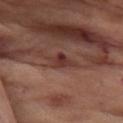Q: Was this lesion biopsied?
A: total-body-photography surveillance lesion; no biopsy
Q: What kind of image is this?
A: 15 mm crop, total-body photography
Q: What is the anatomic site?
A: the front of the torso
Q: What is the lesion's diameter?
A: ~4 mm (longest diameter)
Q: Patient demographics?
A: female, in their mid-50s